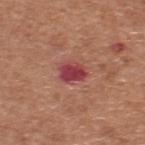Imaged during a routine full-body skin examination; the lesion was not biopsied and no histopathology is available.
A male subject, aged 63 to 67.
Imaged with white-light lighting.
The lesion is located on the upper back.
An algorithmic analysis of the crop reported an area of roughly 6 mm² and two-axis asymmetry of about 0.2. And it measured a normalized border contrast of about 10.5.
A close-up tile cropped from a whole-body skin photograph, about 15 mm across.